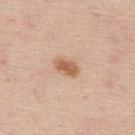Assessment: The lesion was tiled from a total-body skin photograph and was not biopsied. Context: From the upper back. Approximately 3 mm at its widest. This is a white-light tile. An algorithmic analysis of the crop reported a border-irregularity index near 2/10, a within-lesion color-variation index near 3.5/10, and radial color variation of about 1. A male subject, roughly 45 years of age. Cropped from a whole-body photographic skin survey; the tile spans about 15 mm.